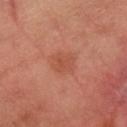follow-up=no biopsy performed (imaged during a skin exam).